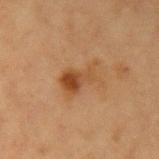diameter: ≈4.5 mm | patient: female, roughly 40 years of age | lighting: cross-polarized illumination | site: the left upper arm | image-analysis metrics: an area of roughly 8.5 mm², an eccentricity of roughly 0.8, and a shape-asymmetry score of about 0.4 (0 = symmetric); a lesion color around L≈42 a*≈20 b*≈33 in CIELAB, about 8 CIELAB-L* units darker than the surrounding skin, and a normalized border contrast of about 7.5; border irregularity of about 5.5 on a 0–10 scale, a color-variation rating of about 8/10, and a peripheral color-asymmetry measure near 3 | acquisition: ~15 mm tile from a whole-body skin photo.